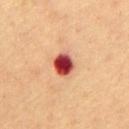No biopsy was performed on this lesion — it was imaged during a full skin examination and was not determined to be concerning. The lesion is located on the chest. A 15 mm crop from a total-body photograph taken for skin-cancer surveillance. The patient is a male in their 60s.